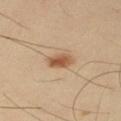Impression:
Imaged during a routine full-body skin examination; the lesion was not biopsied and no histopathology is available.
Background:
The lesion is on the right upper arm. The tile uses cross-polarized illumination. The lesion-visualizer software estimated a lesion area of about 5 mm², a shape eccentricity near 0.8, and two-axis asymmetry of about 0.25. It also reported a mean CIELAB color near L≈54 a*≈20 b*≈34, about 12 CIELAB-L* units darker than the surrounding skin, and a normalized lesion–skin contrast near 8.5. And it measured a nevus-likeness score of about 95/100 and lesion-presence confidence of about 100/100. A roughly 15 mm field-of-view crop from a total-body skin photograph. A male patient approximately 40 years of age.